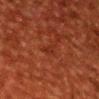No biopsy was performed on this lesion — it was imaged during a full skin examination and was not determined to be concerning.
A male patient, aged 58 to 62.
Cropped from a whole-body photographic skin survey; the tile spans about 15 mm.
The lesion is on the head or neck.
About 2.5 mm across.